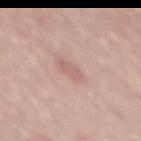This lesion was catalogued during total-body skin photography and was not selected for biopsy.
A 15 mm crop from a total-body photograph taken for skin-cancer surveillance.
From the mid back.
The lesion-visualizer software estimated a lesion area of about 3.5 mm², an eccentricity of roughly 0.8, and two-axis asymmetry of about 0.25. The analysis additionally found a lesion–skin lightness drop of about 7 and a lesion-to-skin contrast of about 4.5 (normalized; higher = more distinct). And it measured a classifier nevus-likeness of about 0/100 and a lesion-detection confidence of about 100/100.
The tile uses white-light illumination.
Longest diameter approximately 2.5 mm.
The patient is a male aged around 60.